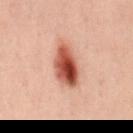The lesion was photographed on a routine skin check and not biopsied; there is no pathology result.